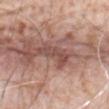This lesion was catalogued during total-body skin photography and was not selected for biopsy. The total-body-photography lesion software estimated a border-irregularity index near 6/10. The lesion's longest dimension is about 4.5 mm. A close-up tile cropped from a whole-body skin photograph, about 15 mm across. A male patient, aged approximately 80. Captured under white-light illumination. The lesion is located on the abdomen.